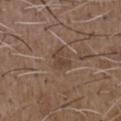No biopsy was performed on this lesion — it was imaged during a full skin examination and was not determined to be concerning.
A male subject, aged 48 to 52.
Measured at roughly 2.5 mm in maximum diameter.
From the chest.
Imaged with white-light lighting.
Cropped from a total-body skin-imaging series; the visible field is about 15 mm.